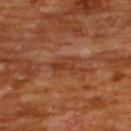workup: total-body-photography surveillance lesion; no biopsy | location: the upper back | image source: total-body-photography crop, ~15 mm field of view | subject: male, about 65 years old.